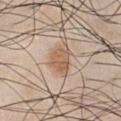This lesion was catalogued during total-body skin photography and was not selected for biopsy.
This image is a 15 mm lesion crop taken from a total-body photograph.
Automated tile analysis of the lesion measured a lesion area of about 6.5 mm², an eccentricity of roughly 0.65, and two-axis asymmetry of about 0.25. And it measured a mean CIELAB color near L≈59 a*≈17 b*≈32 and a lesion-to-skin contrast of about 7.5 (normalized; higher = more distinct).
On the front of the torso.
This is a white-light tile.
About 3 mm across.
The patient is a male in their mid-40s.